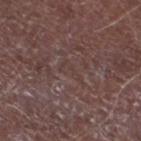<record>
  <biopsy_status>not biopsied; imaged during a skin examination</biopsy_status>
  <site>leg</site>
  <patient>
    <sex>male</sex>
    <age_approx>75</age_approx>
  </patient>
  <automated_metrics>
    <nevus_likeness_0_100>0</nevus_likeness_0_100>
  </automated_metrics>
  <image>
    <source>total-body photography crop</source>
    <field_of_view_mm>15</field_of_view_mm>
  </image>
</record>